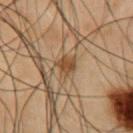Part of a total-body skin-imaging series; this lesion was reviewed on a skin check and was not flagged for biopsy. The recorded lesion diameter is about 3 mm. The subject is a male in their mid-50s. Captured under cross-polarized illumination. Automated image analysis of the tile measured an average lesion color of about L≈39 a*≈14 b*≈28 (CIELAB), a lesion–skin lightness drop of about 9, and a normalized border contrast of about 8. The lesion is located on the chest. Cropped from a total-body skin-imaging series; the visible field is about 15 mm.